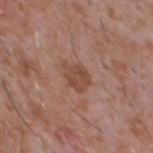Recorded during total-body skin imaging; not selected for excision or biopsy. Located on the chest. The tile uses white-light illumination. Cropped from a total-body skin-imaging series; the visible field is about 15 mm. The lesion's longest dimension is about 3.5 mm. A male patient, about 45 years old.